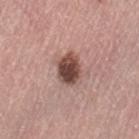| feature | finding |
|---|---|
| biopsy status | total-body-photography surveillance lesion; no biopsy |
| site | the left thigh |
| patient | female, in their mid- to late 60s |
| lesion diameter | ≈4 mm |
| image | total-body-photography crop, ~15 mm field of view |
| automated lesion analysis | an area of roughly 8.5 mm², an eccentricity of roughly 0.65, and two-axis asymmetry of about 0.15; a lesion–skin lightness drop of about 17 and a lesion-to-skin contrast of about 12 (normalized; higher = more distinct); a border-irregularity rating of about 2/10, internal color variation of about 5.5 on a 0–10 scale, and radial color variation of about 2 |